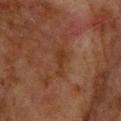Imaged during a routine full-body skin examination; the lesion was not biopsied and no histopathology is available.